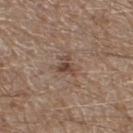The lesion was photographed on a routine skin check and not biopsied; there is no pathology result. Captured under white-light illumination. The lesion-visualizer software estimated an area of roughly 4 mm², an outline eccentricity of about 0.6 (0 = round, 1 = elongated), and a shape-asymmetry score of about 0.5 (0 = symmetric). The subject is a male aged 63–67. Located on the left lower leg. Cropped from a total-body skin-imaging series; the visible field is about 15 mm.